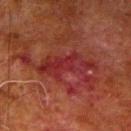<case>
  <patient>
    <sex>male</sex>
    <age_approx>80</age_approx>
  </patient>
  <lighting>cross-polarized</lighting>
  <site>arm</site>
  <lesion_size>
    <long_diameter_mm_approx>7.0</long_diameter_mm_approx>
  </lesion_size>
  <image>
    <source>total-body photography crop</source>
    <field_of_view_mm>15</field_of_view_mm>
  </image>
</case>